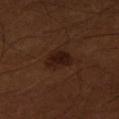No biopsy was performed on this lesion — it was imaged during a full skin examination and was not determined to be concerning.
A roughly 15 mm field-of-view crop from a total-body skin photograph.
This is a cross-polarized tile.
On the right thigh.
An algorithmic analysis of the crop reported a normalized lesion–skin contrast near 9. It also reported internal color variation of about 2.5 on a 0–10 scale and radial color variation of about 0.5. The software also gave a classifier nevus-likeness of about 95/100.
The subject is a male roughly 50 years of age.
Measured at roughly 3.5 mm in maximum diameter.